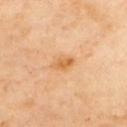Impression:
The lesion was photographed on a routine skin check and not biopsied; there is no pathology result.
Clinical summary:
A region of skin cropped from a whole-body photographic capture, roughly 15 mm wide. The subject is a female aged around 60. The lesion is located on the upper back. Approximately 2.5 mm at its widest.